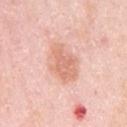Recorded during total-body skin imaging; not selected for excision or biopsy. Cropped from a whole-body photographic skin survey; the tile spans about 15 mm. A female subject about 65 years old. On the left upper arm. Captured under white-light illumination.